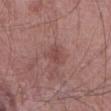Notes:
* lighting: white-light illumination
* automated lesion analysis: a mean CIELAB color near L≈46 a*≈24 b*≈23, about 7 CIELAB-L* units darker than the surrounding skin, and a lesion-to-skin contrast of about 5.5 (normalized; higher = more distinct); a border-irregularity rating of about 3/10; a nevus-likeness score of about 15/100 and a lesion-detection confidence of about 100/100
* location: the abdomen
* patient: male, aged 68 to 72
* diameter: ≈2.5 mm
* imaging modality: ~15 mm crop, total-body skin-cancer survey